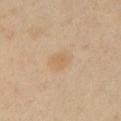The lesion was tiled from a total-body skin photograph and was not biopsied.
Located on the arm.
This image is a 15 mm lesion crop taken from a total-body photograph.
The tile uses cross-polarized illumination.
The lesion's longest dimension is about 3.5 mm.
A male patient about 40 years old.